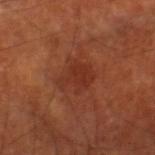This lesion was catalogued during total-body skin photography and was not selected for biopsy. The subject is a male aged approximately 70. A region of skin cropped from a whole-body photographic capture, roughly 15 mm wide. Located on the right thigh. The lesion-visualizer software estimated a lesion area of about 9 mm² and a shape eccentricity near 0.5. The analysis additionally found an average lesion color of about L≈32 a*≈27 b*≈31 (CIELAB) and a lesion-to-skin contrast of about 5.5 (normalized; higher = more distinct). Imaged with cross-polarized lighting.